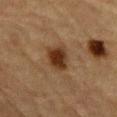{"lesion_size": {"long_diameter_mm_approx": 3.5}, "site": "abdomen", "patient": {"sex": "male", "age_approx": "85+"}, "lighting": "cross-polarized", "image": {"source": "total-body photography crop", "field_of_view_mm": 15}}